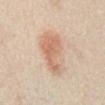The lesion was photographed on a routine skin check and not biopsied; there is no pathology result.